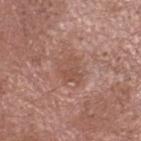Captured during whole-body skin photography for melanoma surveillance; the lesion was not biopsied. Located on the head or neck. A close-up tile cropped from a whole-body skin photograph, about 15 mm across. The subject is a male in their mid- to late 70s.